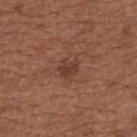notes = imaged on a skin check; not biopsied | tile lighting = white-light illumination | site = the upper back | patient = female, roughly 65 years of age | TBP lesion metrics = an average lesion color of about L≈39 a*≈22 b*≈26 (CIELAB), a lesion–skin lightness drop of about 8, and a normalized lesion–skin contrast near 7 | imaging modality = 15 mm crop, total-body photography | lesion diameter = about 2.5 mm.